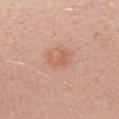Background: A female subject, aged 28–32. The total-body-photography lesion software estimated a footprint of about 4 mm² and a shape-asymmetry score of about 0.45 (0 = symmetric). And it measured border irregularity of about 5.5 on a 0–10 scale, internal color variation of about 0.5 on a 0–10 scale, and peripheral color asymmetry of about 0. The analysis additionally found a classifier nevus-likeness of about 15/100. From the left upper arm. This is a white-light tile. A close-up tile cropped from a whole-body skin photograph, about 15 mm across. Measured at roughly 2.5 mm in maximum diameter.